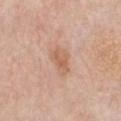Part of a total-body skin-imaging series; this lesion was reviewed on a skin check and was not flagged for biopsy.
Automated tile analysis of the lesion measured an average lesion color of about L≈61 a*≈21 b*≈32 (CIELAB) and a lesion-to-skin contrast of about 6 (normalized; higher = more distinct). It also reported border irregularity of about 3.5 on a 0–10 scale and radial color variation of about 1. And it measured a lesion-detection confidence of about 100/100.
Located on the chest.
The patient is a male in their mid-70s.
Cropped from a total-body skin-imaging series; the visible field is about 15 mm.
This is a white-light tile.